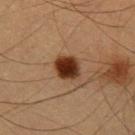The lesion was photographed on a routine skin check and not biopsied; there is no pathology result. A male subject approximately 35 years of age. The lesion is located on the left upper arm. A region of skin cropped from a whole-body photographic capture, roughly 15 mm wide.